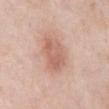* workup · no biopsy performed (imaged during a skin exam)
* image-analysis metrics · an average lesion color of about L≈62 a*≈22 b*≈28 (CIELAB) and a lesion-to-skin contrast of about 6 (normalized; higher = more distinct); border irregularity of about 2 on a 0–10 scale and peripheral color asymmetry of about 1; an automated nevus-likeness rating near 80 out of 100 and a lesion-detection confidence of about 100/100
* anatomic site · the front of the torso
* acquisition · ~15 mm crop, total-body skin-cancer survey
* lesion size · ~4.5 mm (longest diameter)
* patient · male, aged 53–57